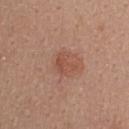| field | value |
|---|---|
| workup | total-body-photography surveillance lesion; no biopsy |